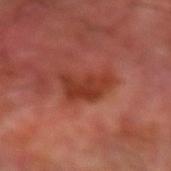follow-up — imaged on a skin check; not biopsied.